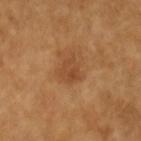biopsy status = imaged on a skin check; not biopsied | tile lighting = cross-polarized illumination | patient = female, aged 53–57 | automated lesion analysis = a lesion area of about 6 mm² and a shape eccentricity near 0.75; internal color variation of about 3.5 on a 0–10 scale and peripheral color asymmetry of about 1.5; a lesion-detection confidence of about 100/100 | body site = the left forearm | imaging modality = 15 mm crop, total-body photography.